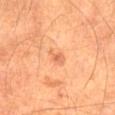The lesion was tiled from a total-body skin photograph and was not biopsied. The patient is a male about 65 years old. The lesion is on the right thigh. A close-up tile cropped from a whole-body skin photograph, about 15 mm across. The total-body-photography lesion software estimated a lesion area of about 3 mm² and an eccentricity of roughly 0.85. And it measured a lesion color around L≈59 a*≈26 b*≈37 in CIELAB and roughly 8 lightness units darker than nearby skin. The analysis additionally found a border-irregularity index near 3.5/10, a color-variation rating of about 2/10, and a peripheral color-asymmetry measure near 0.5. The software also gave an automated nevus-likeness rating near 5 out of 100 and lesion-presence confidence of about 100/100.